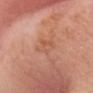{
  "biopsy_status": "not biopsied; imaged during a skin examination",
  "image": {
    "source": "total-body photography crop",
    "field_of_view_mm": 15
  },
  "lesion_size": {
    "long_diameter_mm_approx": 4.0
  },
  "lighting": "white-light",
  "patient": {
    "sex": "male",
    "age_approx": 65
  },
  "site": "head or neck"
}